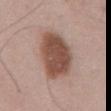Part of a total-body skin-imaging series; this lesion was reviewed on a skin check and was not flagged for biopsy. A male subject, in their mid- to late 50s. A 15 mm close-up tile from a total-body photography series done for melanoma screening. This is a white-light tile. Longest diameter approximately 7 mm.